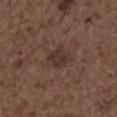Findings:
– workup — imaged on a skin check; not biopsied
– illumination — white-light
– patient — male, aged 48–52
– anatomic site — the right upper arm
– image — total-body-photography crop, ~15 mm field of view
– diameter — ≈2.5 mm